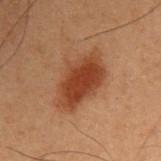Context:
Approximately 6.5 mm at its widest. The patient is a male in their mid- to late 50s. Captured under cross-polarized illumination. A roughly 15 mm field-of-view crop from a total-body skin photograph. From the right upper arm.